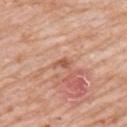Notes:
- biopsy status — no biopsy performed (imaged during a skin exam)
- acquisition — 15 mm crop, total-body photography
- patient — male, in their 80s
- site — the upper back